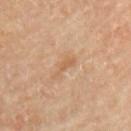| key | value |
|---|---|
| biopsy status | total-body-photography surveillance lesion; no biopsy |
| patient | male, roughly 65 years of age |
| image | ~15 mm tile from a whole-body skin photo |
| illumination | cross-polarized |
| anatomic site | the chest |